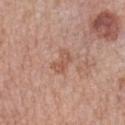* follow-up — imaged on a skin check; not biopsied
* acquisition — 15 mm crop, total-body photography
* image-analysis metrics — a lesion area of about 3.5 mm² and an eccentricity of roughly 0.85; a lesion color around L≈55 a*≈23 b*≈29 in CIELAB, a lesion–skin lightness drop of about 8, and a normalized lesion–skin contrast near 6; border irregularity of about 4 on a 0–10 scale, a within-lesion color-variation index near 0/10, and peripheral color asymmetry of about 0; an automated nevus-likeness rating near 0 out of 100 and lesion-presence confidence of about 100/100
* site — the left forearm
* patient — female, approximately 60 years of age